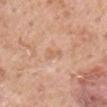biopsy status = catalogued during a skin exam; not biopsied | lesion size = ≈2.5 mm | body site = the right upper arm | illumination = white-light | image = 15 mm crop, total-body photography | image-analysis metrics = a lesion–skin lightness drop of about 6 and a normalized border contrast of about 4.5; a border-irregularity index near 4/10 and radial color variation of about 0; lesion-presence confidence of about 100/100 | patient = male, aged approximately 60.